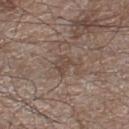{
  "biopsy_status": "not biopsied; imaged during a skin examination",
  "site": "right lower leg",
  "lighting": "white-light",
  "automated_metrics": {
    "cielab_L": 45,
    "cielab_a": 14,
    "cielab_b": 23,
    "vs_skin_darker_L": 7.0,
    "vs_skin_contrast_norm": 5.5,
    "nevus_likeness_0_100": 0
  },
  "image": {
    "source": "total-body photography crop",
    "field_of_view_mm": 15
  },
  "patient": {
    "sex": "male",
    "age_approx": 60
  },
  "lesion_size": {
    "long_diameter_mm_approx": 2.5
  }
}